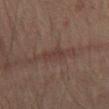workup — total-body-photography surveillance lesion; no biopsy
automated metrics — a lesion area of about 3 mm² and a shape eccentricity near 0.9; an automated nevus-likeness rating near 0 out of 100
lesion size — ≈3 mm
patient — male, aged around 75
site — the right thigh
tile lighting — cross-polarized illumination
image — ~15 mm crop, total-body skin-cancer survey